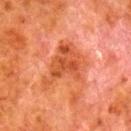Clinical impression:
Captured during whole-body skin photography for melanoma surveillance; the lesion was not biopsied.
Acquisition and patient details:
A male patient approximately 80 years of age. Longest diameter approximately 6 mm. A 15 mm close-up extracted from a 3D total-body photography capture. An algorithmic analysis of the crop reported a mean CIELAB color near L≈43 a*≈28 b*≈35, about 8 CIELAB-L* units darker than the surrounding skin, and a normalized lesion–skin contrast near 7. The analysis additionally found border irregularity of about 6.5 on a 0–10 scale, internal color variation of about 4.5 on a 0–10 scale, and peripheral color asymmetry of about 1.5. On the right lower leg.